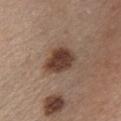{"biopsy_status": "not biopsied; imaged during a skin examination", "patient": {"sex": "female", "age_approx": 45}, "image": {"source": "total-body photography crop", "field_of_view_mm": 15}, "automated_metrics": {"cielab_L": 40, "cielab_a": 18, "cielab_b": 25, "vs_skin_darker_L": 14.0, "vs_skin_contrast_norm": 11.0}, "lesion_size": {"long_diameter_mm_approx": 4.5}, "site": "chest", "lighting": "white-light"}